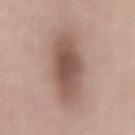  site: mid back
  lesion_size:
    long_diameter_mm_approx: 8.5
  lighting: white-light
  automated_metrics:
    eccentricity: 0.85
    shape_asymmetry: 0.1
    nevus_likeness_0_100: 65
    lesion_detection_confidence_0_100: 100
  image:
    source: total-body photography crop
    field_of_view_mm: 15
  patient:
    sex: male
    age_approx: 70